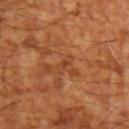Q: Is there a histopathology result?
A: no biopsy performed (imaged during a skin exam)
Q: What kind of image is this?
A: ~15 mm tile from a whole-body skin photo
Q: Lesion location?
A: the left upper arm
Q: Who is the patient?
A: approximately 65 years of age